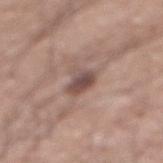Recorded during total-body skin imaging; not selected for excision or biopsy. The lesion is located on the abdomen. Captured under white-light illumination. Cropped from a total-body skin-imaging series; the visible field is about 15 mm. A male subject, approximately 75 years of age. Measured at roughly 3 mm in maximum diameter.